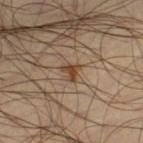The lesion was tiled from a total-body skin photograph and was not biopsied.
On the left thigh.
Cropped from a whole-body photographic skin survey; the tile spans about 15 mm.
Imaged with cross-polarized lighting.
A male patient, aged approximately 45.
About 2 mm across.
An algorithmic analysis of the crop reported a footprint of about 2.5 mm². It also reported a mean CIELAB color near L≈41 a*≈18 b*≈30, a lesion–skin lightness drop of about 11, and a normalized lesion–skin contrast near 10. And it measured a border-irregularity rating of about 5.5/10, internal color variation of about 0 on a 0–10 scale, and a peripheral color-asymmetry measure near 0.